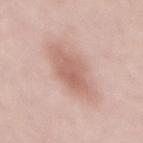Recorded during total-body skin imaging; not selected for excision or biopsy.
On the mid back.
A close-up tile cropped from a whole-body skin photograph, about 15 mm across.
The lesion-visualizer software estimated a footprint of about 17 mm², a shape eccentricity near 0.85, and a symmetry-axis asymmetry near 0.25. It also reported a border-irregularity index near 3/10, a color-variation rating of about 3/10, and peripheral color asymmetry of about 1.
This is a white-light tile.
Measured at roughly 6.5 mm in maximum diameter.
The patient is a female about 60 years old.